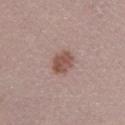<case>
<biopsy_status>not biopsied; imaged during a skin examination</biopsy_status>
<patient>
  <sex>female</sex>
  <age_approx>40</age_approx>
</patient>
<lesion_size>
  <long_diameter_mm_approx>3.0</long_diameter_mm_approx>
</lesion_size>
<image>
  <source>total-body photography crop</source>
  <field_of_view_mm>15</field_of_view_mm>
</image>
<lighting>white-light</lighting>
<site>leg</site>
</case>